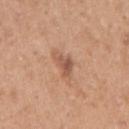Q: Was a biopsy performed?
A: catalogued during a skin exam; not biopsied
Q: What is the imaging modality?
A: total-body-photography crop, ~15 mm field of view
Q: What lighting was used for the tile?
A: white-light
Q: What is the anatomic site?
A: the right upper arm
Q: What did automated image analysis measure?
A: a shape eccentricity near 0.7; a lesion color around L≈55 a*≈22 b*≈31 in CIELAB, a lesion–skin lightness drop of about 10, and a lesion-to-skin contrast of about 6.5 (normalized; higher = more distinct); a border-irregularity index near 5/10 and peripheral color asymmetry of about 1.5
Q: What are the patient's age and sex?
A: female, about 40 years old